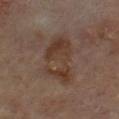workup = catalogued during a skin exam; not biopsied
patient = male, aged around 70
acquisition = ~15 mm tile from a whole-body skin photo
body site = the front of the torso
tile lighting = cross-polarized illumination
TBP lesion metrics = roughly 7 lightness units darker than nearby skin and a normalized border contrast of about 7